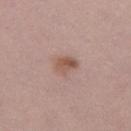diameter: ~3 mm (longest diameter) | image: 15 mm crop, total-body photography | subject: female, aged approximately 20 | body site: the left thigh | lighting: white-light.